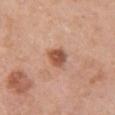Assessment: Part of a total-body skin-imaging series; this lesion was reviewed on a skin check and was not flagged for biopsy. Image and clinical context: A 15 mm close-up extracted from a 3D total-body photography capture. A female subject, aged around 60. Imaged with white-light lighting. From the chest.